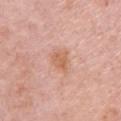workup = no biopsy performed (imaged during a skin exam)
anatomic site = the right upper arm
lesion size = about 2.5 mm
subject = female, aged around 65
tile lighting = white-light
image source = total-body-photography crop, ~15 mm field of view